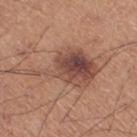biopsy_status: not biopsied; imaged during a skin examination
patient:
  sex: male
  age_approx: 55
image:
  source: total-body photography crop
  field_of_view_mm: 15
automated_metrics:
  eccentricity: 0.85
  shape_asymmetry: 0.5
  cielab_L: 48
  cielab_a: 21
  cielab_b: 26
  vs_skin_darker_L: 11.0
site: right thigh
lighting: white-light